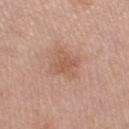No biopsy was performed on this lesion — it was imaged during a full skin examination and was not determined to be concerning. The total-body-photography lesion software estimated a border-irregularity index near 3/10, internal color variation of about 2.5 on a 0–10 scale, and a peripheral color-asymmetry measure near 0.5. This image is a 15 mm lesion crop taken from a total-body photograph. Located on the left lower leg. Imaged with white-light lighting. The subject is a female in their mid-60s.